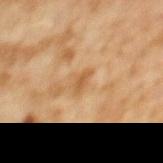<lesion>
  <biopsy_status>not biopsied; imaged during a skin examination</biopsy_status>
  <lesion_size>
    <long_diameter_mm_approx>2.5</long_diameter_mm_approx>
  </lesion_size>
  <lighting>cross-polarized</lighting>
  <image>
    <source>total-body photography crop</source>
    <field_of_view_mm>15</field_of_view_mm>
  </image>
  <site>upper back</site>
  <patient>
    <sex>female</sex>
    <age_approx>60</age_approx>
  </patient>
  <automated_metrics>
    <area_mm2_approx>3.0</area_mm2_approx>
    <eccentricity>0.85</eccentricity>
    <shape_asymmetry>0.35</shape_asymmetry>
    <border_irregularity_0_10>3.5</border_irregularity_0_10>
    <nevus_likeness_0_100>0</nevus_likeness_0_100>
    <lesion_detection_confidence_0_100>100</lesion_detection_confidence_0_100>
  </automated_metrics>
</lesion>